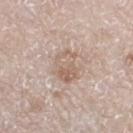A male subject aged approximately 80.
Captured under white-light illumination.
The lesion is located on the left lower leg.
A close-up tile cropped from a whole-body skin photograph, about 15 mm across.
About 4.5 mm across.